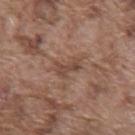No biopsy was performed on this lesion — it was imaged during a full skin examination and was not determined to be concerning. Measured at roughly 3 mm in maximum diameter. A 15 mm crop from a total-body photograph taken for skin-cancer surveillance. The tile uses white-light illumination. The patient is a male aged approximately 75. The lesion is located on the chest.